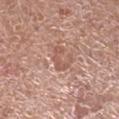| feature | finding |
|---|---|
| workup | no biopsy performed (imaged during a skin exam) |
| patient | male, aged approximately 80 |
| site | the leg |
| lighting | white-light illumination |
| imaging modality | 15 mm crop, total-body photography |